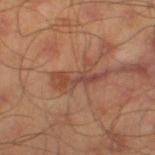{
  "biopsy_status": "not biopsied; imaged during a skin examination",
  "image": {
    "source": "total-body photography crop",
    "field_of_view_mm": 15
  },
  "lighting": "cross-polarized",
  "lesion_size": {
    "long_diameter_mm_approx": 6.0
  },
  "site": "right thigh",
  "patient": {
    "sex": "male",
    "age_approx": 45
  }
}